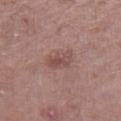Assessment:
Imaged during a routine full-body skin examination; the lesion was not biopsied and no histopathology is available.
Background:
Longest diameter approximately 3.5 mm. Cropped from a total-body skin-imaging series; the visible field is about 15 mm. The lesion is located on the right thigh. This is a white-light tile. A male subject, in their 70s.